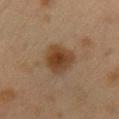Q: Was a biopsy performed?
A: no biopsy performed (imaged during a skin exam)
Q: What are the patient's age and sex?
A: female, aged 38 to 42
Q: What is the lesion's diameter?
A: about 4 mm
Q: How was this image acquired?
A: ~15 mm tile from a whole-body skin photo
Q: Where on the body is the lesion?
A: the chest